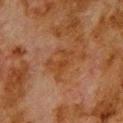Recorded during total-body skin imaging; not selected for excision or biopsy. Measured at roughly 3 mm in maximum diameter. The lesion is located on the upper back. A male patient, aged 78–82. A close-up tile cropped from a whole-body skin photograph, about 15 mm across.